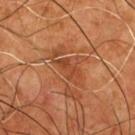* follow-up · total-body-photography surveillance lesion; no biopsy
* size · ~2.5 mm (longest diameter)
* image source · total-body-photography crop, ~15 mm field of view
* anatomic site · the chest
* subject · male, roughly 55 years of age
* automated lesion analysis · a lesion area of about 3 mm², an outline eccentricity of about 0.85 (0 = round, 1 = elongated), and two-axis asymmetry of about 0.45; a lesion–skin lightness drop of about 6 and a normalized border contrast of about 5.5; a nevus-likeness score of about 0/100